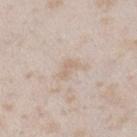The lesion was tiled from a total-body skin photograph and was not biopsied. A roughly 15 mm field-of-view crop from a total-body skin photograph. The subject is a female aged 23 to 27. The lesion is on the left lower leg.